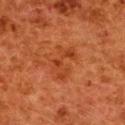Findings:
• notes · catalogued during a skin exam; not biopsied
• tile lighting · cross-polarized
• lesion size · ~4.5 mm (longest diameter)
• location · the upper back
• acquisition · 15 mm crop, total-body photography
• subject · female, roughly 50 years of age